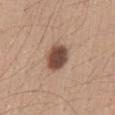Q: Is there a histopathology result?
A: catalogued during a skin exam; not biopsied
Q: What is the anatomic site?
A: the front of the torso
Q: What did automated image analysis measure?
A: a lesion area of about 9.5 mm², an eccentricity of roughly 0.55, and a shape-asymmetry score of about 0.15 (0 = symmetric); a lesion-detection confidence of about 100/100
Q: Illumination type?
A: white-light illumination
Q: Lesion size?
A: about 3.5 mm
Q: Patient demographics?
A: male, aged approximately 30
Q: How was this image acquired?
A: ~15 mm tile from a whole-body skin photo